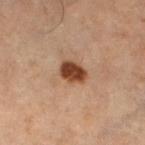Captured during whole-body skin photography for melanoma surveillance; the lesion was not biopsied. A female patient, aged 48 to 52. Measured at roughly 3 mm in maximum diameter. A 15 mm close-up extracted from a 3D total-body photography capture. Located on the left thigh. Automated image analysis of the tile measured a lesion-to-skin contrast of about 12.5 (normalized; higher = more distinct). The software also gave lesion-presence confidence of about 100/100. Captured under cross-polarized illumination.